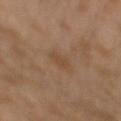The lesion was photographed on a routine skin check and not biopsied; there is no pathology result.
This is a cross-polarized tile.
Longest diameter approximately 2.5 mm.
A roughly 15 mm field-of-view crop from a total-body skin photograph.
On the left upper arm.
The patient is a male roughly 30 years of age.
The lesion-visualizer software estimated a shape eccentricity near 0.8 and two-axis asymmetry of about 0.3. The analysis additionally found a mean CIELAB color near L≈44 a*≈16 b*≈30, roughly 5 lightness units darker than nearby skin, and a lesion-to-skin contrast of about 5 (normalized; higher = more distinct). The analysis additionally found a border-irregularity rating of about 3/10 and peripheral color asymmetry of about 0. And it measured a classifier nevus-likeness of about 5/100.